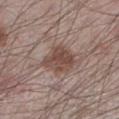Clinical impression:
Imaged during a routine full-body skin examination; the lesion was not biopsied and no histopathology is available.